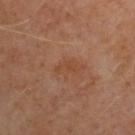The lesion was tiled from a total-body skin photograph and was not biopsied.
From the upper back.
This is a cross-polarized tile.
A female patient, aged 58–62.
Automated image analysis of the tile measured a lesion color around L≈44 a*≈23 b*≈32 in CIELAB and a lesion-to-skin contrast of about 5 (normalized; higher = more distinct). The analysis additionally found a border-irregularity index near 4.5/10, a color-variation rating of about 0.5/10, and a peripheral color-asymmetry measure near 0.
Cropped from a whole-body photographic skin survey; the tile spans about 15 mm.